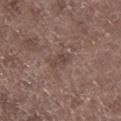This lesion was catalogued during total-body skin photography and was not selected for biopsy.
A close-up tile cropped from a whole-body skin photograph, about 15 mm across.
The lesion-visualizer software estimated a lesion area of about 3 mm², an outline eccentricity of about 0.85 (0 = round, 1 = elongated), and two-axis asymmetry of about 0.25. The software also gave a border-irregularity index near 3/10 and peripheral color asymmetry of about 0. It also reported a classifier nevus-likeness of about 0/100 and a detector confidence of about 95 out of 100 that the crop contains a lesion.
Imaged with white-light lighting.
From the left lower leg.
A male patient aged 68 to 72.
Longest diameter approximately 2.5 mm.